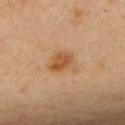Part of a total-body skin-imaging series; this lesion was reviewed on a skin check and was not flagged for biopsy. The lesion is on the upper back. Captured under cross-polarized illumination. A lesion tile, about 15 mm wide, cut from a 3D total-body photograph. A female subject approximately 45 years of age. Approximately 3.5 mm at its widest.